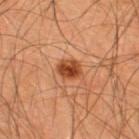This lesion was catalogued during total-body skin photography and was not selected for biopsy. A lesion tile, about 15 mm wide, cut from a 3D total-body photograph. The subject is a male aged 43 to 47. On the upper back. This is a cross-polarized tile.